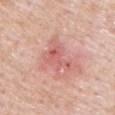A region of skin cropped from a whole-body photographic capture, roughly 15 mm wide. The patient is a male aged around 55. An algorithmic analysis of the crop reported a normalized lesion–skin contrast near 5.5. The software also gave an automated nevus-likeness rating near 0 out of 100 and a detector confidence of about 100 out of 100 that the crop contains a lesion. About 5 mm across. The lesion is located on the chest.